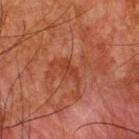Q: Was this lesion biopsied?
A: total-body-photography surveillance lesion; no biopsy
Q: What kind of image is this?
A: total-body-photography crop, ~15 mm field of view
Q: Lesion size?
A: ≈2.5 mm
Q: What are the patient's age and sex?
A: male, aged approximately 65
Q: How was the tile lit?
A: cross-polarized
Q: What is the anatomic site?
A: the upper back